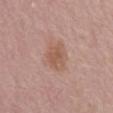notes: total-body-photography surveillance lesion; no biopsy
tile lighting: white-light illumination
patient: female, in their mid- to late 60s
TBP lesion metrics: an eccentricity of roughly 0.6 and a shape-asymmetry score of about 0.2 (0 = symmetric); a border-irregularity rating of about 2/10, internal color variation of about 3 on a 0–10 scale, and peripheral color asymmetry of about 1
size: ~3.5 mm (longest diameter)
location: the back
imaging modality: 15 mm crop, total-body photography